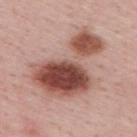{
  "biopsy_status": "not biopsied; imaged during a skin examination",
  "image": {
    "source": "total-body photography crop",
    "field_of_view_mm": 15
  },
  "patient": {
    "sex": "male",
    "age_approx": 50
  },
  "site": "upper back"
}